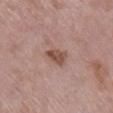This lesion was catalogued during total-body skin photography and was not selected for biopsy. This is a white-light tile. Located on the right lower leg. The patient is a female aged 68 to 72. A region of skin cropped from a whole-body photographic capture, roughly 15 mm wide. Approximately 3 mm at its widest.